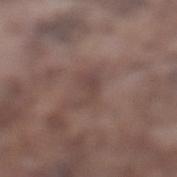Recorded during total-body skin imaging; not selected for excision or biopsy. The patient is a male aged around 75. Cropped from a total-body skin-imaging series; the visible field is about 15 mm. Located on the left lower leg.